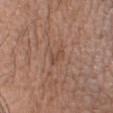Impression:
Part of a total-body skin-imaging series; this lesion was reviewed on a skin check and was not flagged for biopsy.
Acquisition and patient details:
A roughly 15 mm field-of-view crop from a total-body skin photograph. The tile uses white-light illumination. Located on the head or neck. The patient is a female about 50 years old. The total-body-photography lesion software estimated a footprint of about 2.5 mm² and a shape eccentricity near 0.9. The software also gave a lesion color around L≈47 a*≈20 b*≈28 in CIELAB, about 6 CIELAB-L* units darker than the surrounding skin, and a normalized lesion–skin contrast near 5. It also reported a classifier nevus-likeness of about 0/100 and a detector confidence of about 95 out of 100 that the crop contains a lesion.